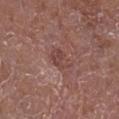biopsy_status: not biopsied; imaged during a skin examination
image:
  source: total-body photography crop
  field_of_view_mm: 15
patient:
  sex: male
  age_approx: 75
lighting: white-light
lesion_size:
  long_diameter_mm_approx: 3.0
site: right lower leg